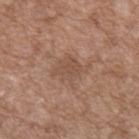Q: Was a biopsy performed?
A: total-body-photography surveillance lesion; no biopsy
Q: What kind of image is this?
A: total-body-photography crop, ~15 mm field of view
Q: Where on the body is the lesion?
A: the left upper arm
Q: Who is the patient?
A: male, about 50 years old